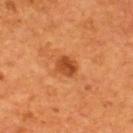Captured during whole-body skin photography for melanoma surveillance; the lesion was not biopsied. The patient is a male roughly 55 years of age. This is a cross-polarized tile. A close-up tile cropped from a whole-body skin photograph, about 15 mm across. Approximately 2.5 mm at its widest. On the back.